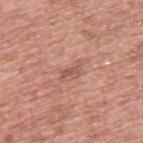Q: Was this lesion biopsied?
A: catalogued during a skin exam; not biopsied
Q: Lesion size?
A: about 3 mm
Q: What are the patient's age and sex?
A: male, aged 53 to 57
Q: Automated lesion metrics?
A: about 9 CIELAB-L* units darker than the surrounding skin and a normalized border contrast of about 6; a border-irregularity index near 6.5/10 and a peripheral color-asymmetry measure near 0
Q: What kind of image is this?
A: total-body-photography crop, ~15 mm field of view
Q: Where on the body is the lesion?
A: the back
Q: What lighting was used for the tile?
A: white-light illumination